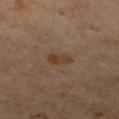{
  "biopsy_status": "not biopsied; imaged during a skin examination",
  "site": "right lower leg",
  "patient": {
    "sex": "male",
    "age_approx": 60
  },
  "lesion_size": {
    "long_diameter_mm_approx": 2.5
  },
  "automated_metrics": {
    "eccentricity": 0.85,
    "shape_asymmetry": 0.35,
    "cielab_L": 35,
    "cielab_a": 15,
    "cielab_b": 27,
    "vs_skin_darker_L": 7.0,
    "vs_skin_contrast_norm": 7.5,
    "nevus_likeness_0_100": 20,
    "lesion_detection_confidence_0_100": 100
  },
  "image": {
    "source": "total-body photography crop",
    "field_of_view_mm": 15
  }
}